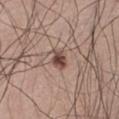Impression:
The lesion was photographed on a routine skin check and not biopsied; there is no pathology result.
Acquisition and patient details:
The lesion's longest dimension is about 3 mm. A 15 mm crop from a total-body photograph taken for skin-cancer surveillance. A male subject, aged around 70. An algorithmic analysis of the crop reported a footprint of about 5 mm² and an eccentricity of roughly 0.7. And it measured an average lesion color of about L≈48 a*≈18 b*≈24 (CIELAB), about 13 CIELAB-L* units darker than the surrounding skin, and a normalized border contrast of about 9.5. The software also gave an automated nevus-likeness rating near 95 out of 100 and a lesion-detection confidence of about 100/100. The lesion is located on the abdomen.